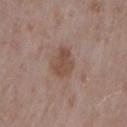Imaged during a routine full-body skin examination; the lesion was not biopsied and no histopathology is available.
A 15 mm crop from a total-body photograph taken for skin-cancer surveillance.
The patient is a male aged 53–57.
From the right upper arm.